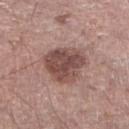{"biopsy_status": "not biopsied; imaged during a skin examination", "lesion_size": {"long_diameter_mm_approx": 5.0}, "image": {"source": "total-body photography crop", "field_of_view_mm": 15}, "patient": {"sex": "male", "age_approx": 55}, "site": "right lower leg", "lighting": "white-light"}